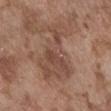The lesion was photographed on a routine skin check and not biopsied; there is no pathology result. A male subject aged around 75. A lesion tile, about 15 mm wide, cut from a 3D total-body photograph. On the abdomen.